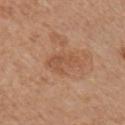follow-up: imaged on a skin check; not biopsied
patient: female, roughly 55 years of age
image: total-body-photography crop, ~15 mm field of view
illumination: white-light
site: the arm